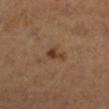Recorded during total-body skin imaging; not selected for excision or biopsy. The lesion is located on the right lower leg. The recorded lesion diameter is about 2.5 mm. Captured under cross-polarized illumination. An algorithmic analysis of the crop reported a footprint of about 3.5 mm² and a symmetry-axis asymmetry near 0.4. And it measured roughly 9 lightness units darker than nearby skin and a lesion-to-skin contrast of about 8 (normalized; higher = more distinct). It also reported border irregularity of about 4 on a 0–10 scale, a within-lesion color-variation index near 1.5/10, and peripheral color asymmetry of about 0.5. The patient is a female roughly 55 years of age. Cropped from a whole-body photographic skin survey; the tile spans about 15 mm.